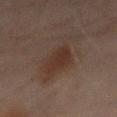workup: catalogued during a skin exam; not biopsied | anatomic site: the mid back | image-analysis metrics: a mean CIELAB color near L≈26 a*≈13 b*≈19, a lesion–skin lightness drop of about 6, and a normalized border contrast of about 7; an automated nevus-likeness rating near 75 out of 100 and lesion-presence confidence of about 100/100 | subject: male, roughly 45 years of age | size: ~8.5 mm (longest diameter) | image source: ~15 mm crop, total-body skin-cancer survey.